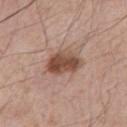The lesion was tiled from a total-body skin photograph and was not biopsied.
The lesion is located on the chest.
Longest diameter approximately 4.5 mm.
A male patient, aged approximately 55.
The total-body-photography lesion software estimated a footprint of about 10 mm² and an outline eccentricity of about 0.7 (0 = round, 1 = elongated). The analysis additionally found an automated nevus-likeness rating near 90 out of 100 and lesion-presence confidence of about 100/100.
A 15 mm close-up extracted from a 3D total-body photography capture.
The tile uses white-light illumination.